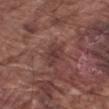follow-up: imaged on a skin check; not biopsied.